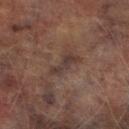Impression:
No biopsy was performed on this lesion — it was imaged during a full skin examination and was not determined to be concerning.
Acquisition and patient details:
Measured at roughly 3.5 mm in maximum diameter. The subject is a male aged approximately 75. The total-body-photography lesion software estimated a footprint of about 4.5 mm², an outline eccentricity of about 0.95 (0 = round, 1 = elongated), and two-axis asymmetry of about 0.35. It also reported a classifier nevus-likeness of about 0/100. A roughly 15 mm field-of-view crop from a total-body skin photograph. On the right lower leg. The tile uses cross-polarized illumination.